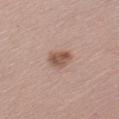- biopsy status: total-body-photography surveillance lesion; no biopsy
- location: the left upper arm
- image source: ~15 mm crop, total-body skin-cancer survey
- lesion diameter: ≈3 mm
- subject: female, aged 43–47
- illumination: white-light